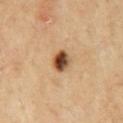notes: catalogued during a skin exam; not biopsied
body site: the chest
size: about 2.5 mm
subject: male, aged 68 to 72
acquisition: ~15 mm tile from a whole-body skin photo
image-analysis metrics: a footprint of about 5.5 mm², an eccentricity of roughly 0.45, and a symmetry-axis asymmetry near 0.1; roughly 18 lightness units darker than nearby skin; a border-irregularity index near 1/10, a within-lesion color-variation index near 9.5/10, and a peripheral color-asymmetry measure near 3